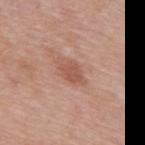Q: Was this lesion biopsied?
A: catalogued during a skin exam; not biopsied
Q: Who is the patient?
A: male, about 55 years old
Q: How large is the lesion?
A: about 3 mm
Q: How was this image acquired?
A: total-body-photography crop, ~15 mm field of view
Q: Lesion location?
A: the mid back
Q: How was the tile lit?
A: white-light illumination